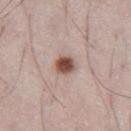biopsy status: no biopsy performed (imaged during a skin exam) | patient: male, aged around 50 | location: the leg | image: ~15 mm tile from a whole-body skin photo | size: about 2.5 mm.